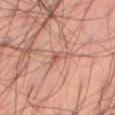Q: Was a biopsy performed?
A: catalogued during a skin exam; not biopsied
Q: How large is the lesion?
A: ~2.5 mm (longest diameter)
Q: Illumination type?
A: cross-polarized illumination
Q: Lesion location?
A: the right thigh
Q: Patient demographics?
A: male, about 45 years old
Q: How was this image acquired?
A: ~15 mm tile from a whole-body skin photo